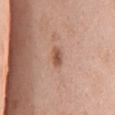<lesion>
  <image>
    <source>total-body photography crop</source>
    <field_of_view_mm>15</field_of_view_mm>
  </image>
  <patient>
    <sex>female</sex>
    <age_approx>50</age_approx>
  </patient>
  <site>mid back</site>
</lesion>